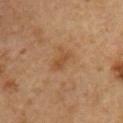– notes: no biopsy performed (imaged during a skin exam)
– subject: male, in their mid- to late 50s
– body site: the front of the torso
– diameter: ~2.5 mm (longest diameter)
– imaging modality: ~15 mm crop, total-body skin-cancer survey
– lighting: cross-polarized illumination